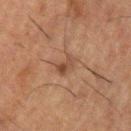biopsy status=catalogued during a skin exam; not biopsied
automated metrics=an average lesion color of about L≈36 a*≈16 b*≈25 (CIELAB), about 7 CIELAB-L* units darker than the surrounding skin, and a normalized border contrast of about 6.5; an automated nevus-likeness rating near 75 out of 100 and lesion-presence confidence of about 100/100
site=the left upper arm
illumination=cross-polarized
size=about 3 mm
patient=male, aged 48–52
acquisition=~15 mm crop, total-body skin-cancer survey